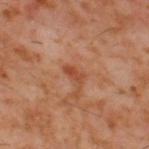No biopsy was performed on this lesion — it was imaged during a full skin examination and was not determined to be concerning. On the upper back. This image is a 15 mm lesion crop taken from a total-body photograph. A male subject, approximately 60 years of age. Captured under cross-polarized illumination.